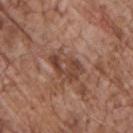follow-up: no biopsy performed (imaged during a skin exam)
site: the chest
subject: male, roughly 70 years of age
acquisition: ~15 mm crop, total-body skin-cancer survey
image-analysis metrics: an average lesion color of about L≈44 a*≈21 b*≈27 (CIELAB) and a normalized border contrast of about 7.5
lighting: white-light illumination